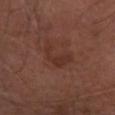Impression:
This lesion was catalogued during total-body skin photography and was not selected for biopsy.
Acquisition and patient details:
The lesion-visualizer software estimated a footprint of about 6 mm², an eccentricity of roughly 0.9, and two-axis asymmetry of about 0.65. The software also gave a border-irregularity index near 7.5/10, internal color variation of about 1.5 on a 0–10 scale, and radial color variation of about 0.5. This image is a 15 mm lesion crop taken from a total-body photograph. From the head or neck. Approximately 4.5 mm at its widest. A male patient aged 73–77. Captured under white-light illumination.